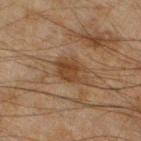| field | value |
|---|---|
| acquisition | total-body-photography crop, ~15 mm field of view |
| body site | the leg |
| subject | male, in their mid-40s |